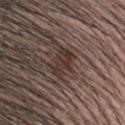This lesion was catalogued during total-body skin photography and was not selected for biopsy.